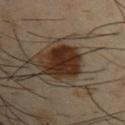Findings:
* imaging modality — ~15 mm tile from a whole-body skin photo
* lighting — cross-polarized
* diameter — ~5.5 mm (longest diameter)
* location — the left upper arm
* image-analysis metrics — a lesion area of about 15 mm² and a shape-asymmetry score of about 0.2 (0 = symmetric); a mean CIELAB color near L≈27 a*≈16 b*≈25, about 15 CIELAB-L* units darker than the surrounding skin, and a normalized lesion–skin contrast near 14.5; a detector confidence of about 100 out of 100 that the crop contains a lesion
* patient — male, aged 38–42